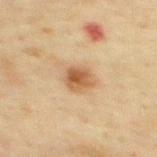No biopsy was performed on this lesion — it was imaged during a full skin examination and was not determined to be concerning. A male patient aged approximately 45. This is a cross-polarized tile. Approximately 3 mm at its widest. This image is a 15 mm lesion crop taken from a total-body photograph. The lesion is located on the mid back.